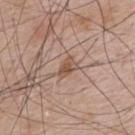Assessment:
Part of a total-body skin-imaging series; this lesion was reviewed on a skin check and was not flagged for biopsy.
Clinical summary:
On the upper back. A roughly 15 mm field-of-view crop from a total-body skin photograph. Automated image analysis of the tile measured border irregularity of about 3.5 on a 0–10 scale. And it measured a classifier nevus-likeness of about 15/100 and a lesion-detection confidence of about 100/100. Imaged with white-light lighting. A male patient in their mid-60s.